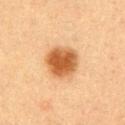| feature | finding |
|---|---|
| workup | total-body-photography surveillance lesion; no biopsy |
| image | ~15 mm tile from a whole-body skin photo |
| diameter | ≈4 mm |
| patient | male, aged approximately 55 |
| anatomic site | the arm |
| illumination | cross-polarized |